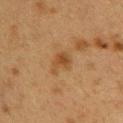A roughly 15 mm field-of-view crop from a total-body skin photograph.
The lesion is on the upper back.
The subject is a female aged 38–42.
This is a cross-polarized tile.
Automated image analysis of the tile measured a footprint of about 4.5 mm², an eccentricity of roughly 0.7, and two-axis asymmetry of about 0.45. The software also gave roughly 7 lightness units darker than nearby skin and a normalized border contrast of about 7. The analysis additionally found an automated nevus-likeness rating near 20 out of 100 and a detector confidence of about 100 out of 100 that the crop contains a lesion.